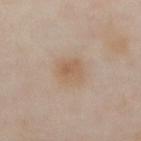Notes:
* workup: total-body-photography surveillance lesion; no biopsy
* site: the abdomen
* subject: male, aged 63 to 67
* image: total-body-photography crop, ~15 mm field of view